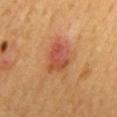The lesion was photographed on a routine skin check and not biopsied; there is no pathology result. The lesion's longest dimension is about 4 mm. On the mid back. Automated tile analysis of the lesion measured an area of roughly 11 mm². And it measured a lesion color around L≈50 a*≈28 b*≈34 in CIELAB, about 9 CIELAB-L* units darker than the surrounding skin, and a normalized border contrast of about 7. And it measured a border-irregularity rating of about 3/10, internal color variation of about 5.5 on a 0–10 scale, and peripheral color asymmetry of about 1.5. The analysis additionally found a classifier nevus-likeness of about 30/100 and a lesion-detection confidence of about 100/100. This is a cross-polarized tile. The patient is a male aged 58–62. A 15 mm close-up tile from a total-body photography series done for melanoma screening.